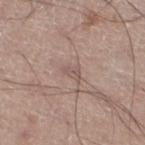follow-up: no biopsy performed (imaged during a skin exam) | patient: male, about 50 years old | image: total-body-photography crop, ~15 mm field of view | site: the leg.